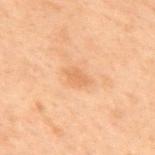biopsy status: imaged on a skin check; not biopsied | location: the mid back | lesion size: about 2.5 mm | subject: male, aged approximately 70 | automated metrics: an area of roughly 3.5 mm², an eccentricity of roughly 0.8, and a shape-asymmetry score of about 0.25 (0 = symmetric); border irregularity of about 2.5 on a 0–10 scale and a within-lesion color-variation index near 1/10 | lighting: cross-polarized illumination | acquisition: ~15 mm crop, total-body skin-cancer survey.